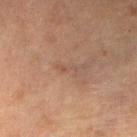A lesion tile, about 15 mm wide, cut from a 3D total-body photograph. Captured under cross-polarized illumination. The subject is a female approximately 55 years of age. Approximately 3 mm at its widest. The total-body-photography lesion software estimated a shape eccentricity near 0.95 and two-axis asymmetry of about 0.4. The software also gave an automated nevus-likeness rating near 0 out of 100 and a detector confidence of about 95 out of 100 that the crop contains a lesion. The lesion is on the left lower leg.